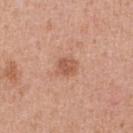notes = no biopsy performed (imaged during a skin exam); lesion size = about 2.5 mm; subject = male, aged 53 to 57; tile lighting = white-light; location = the front of the torso; imaging modality = 15 mm crop, total-body photography.